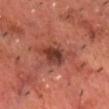follow-up = no biopsy performed (imaged during a skin exam) | site = the chest | subject = male, aged 68–72 | acquisition = ~15 mm crop, total-body skin-cancer survey | lighting = cross-polarized.